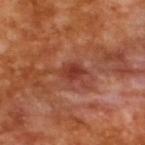A male patient, about 65 years old.
Captured under cross-polarized illumination.
Approximately 3 mm at its widest.
A 15 mm crop from a total-body photograph taken for skin-cancer surveillance.
The lesion-visualizer software estimated a lesion color around L≈38 a*≈29 b*≈30 in CIELAB and a normalized border contrast of about 7.5. It also reported a border-irregularity index near 2/10, internal color variation of about 2.5 on a 0–10 scale, and radial color variation of about 0.5.